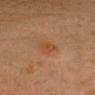- workup · total-body-photography surveillance lesion; no biopsy
- patient · female, in their 20s
- location · the head or neck
- imaging modality · ~15 mm tile from a whole-body skin photo
- diameter · ≈2.5 mm